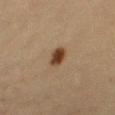<case>
<biopsy_status>not biopsied; imaged during a skin examination</biopsy_status>
<site>leg</site>
<patient>
  <sex>female</sex>
  <age_approx>65</age_approx>
</patient>
<lighting>cross-polarized</lighting>
<lesion_size>
  <long_diameter_mm_approx>3.0</long_diameter_mm_approx>
</lesion_size>
<automated_metrics>
  <cielab_L>35</cielab_L>
  <cielab_a>16</cielab_a>
  <cielab_b>28</cielab_b>
  <vs_skin_darker_L>13.0</vs_skin_darker_L>
  <vs_skin_contrast_norm>11.5</vs_skin_contrast_norm>
  <border_irregularity_0_10>2.0</border_irregularity_0_10>
  <nevus_likeness_0_100>100</nevus_likeness_0_100>
  <lesion_detection_confidence_0_100>100</lesion_detection_confidence_0_100>
</automated_metrics>
<image>
  <source>total-body photography crop</source>
  <field_of_view_mm>15</field_of_view_mm>
</image>
</case>